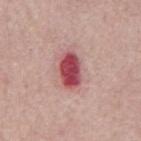Assessment:
Imaged during a routine full-body skin examination; the lesion was not biopsied and no histopathology is available.
Image and clinical context:
A male subject in their mid-60s. Located on the back. Imaged with white-light lighting. Measured at roughly 4 mm in maximum diameter. A 15 mm close-up extracted from a 3D total-body photography capture.